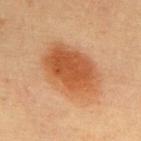Captured during whole-body skin photography for melanoma surveillance; the lesion was not biopsied.
A female subject, in their mid- to late 60s.
Imaged with cross-polarized lighting.
The lesion is located on the upper back.
A close-up tile cropped from a whole-body skin photograph, about 15 mm across.
Measured at roughly 7.5 mm in maximum diameter.
Automated image analysis of the tile measured an area of roughly 29 mm², a shape eccentricity near 0.75, and a shape-asymmetry score of about 0.1 (0 = symmetric). The analysis additionally found a classifier nevus-likeness of about 100/100 and lesion-presence confidence of about 100/100.